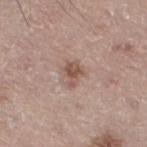Assessment:
Recorded during total-body skin imaging; not selected for excision or biopsy.
Acquisition and patient details:
Automated image analysis of the tile measured a lesion color around L≈52 a*≈18 b*≈25 in CIELAB, about 11 CIELAB-L* units darker than the surrounding skin, and a normalized lesion–skin contrast near 8. And it measured border irregularity of about 4 on a 0–10 scale and a color-variation rating of about 2.5/10. Longest diameter approximately 2.5 mm. This is a white-light tile. A male patient, in their 70s. Located on the right leg. A lesion tile, about 15 mm wide, cut from a 3D total-body photograph.